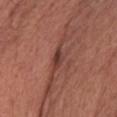follow-up = no biopsy performed (imaged during a skin exam) | diameter = ≈3 mm | imaging modality = ~15 mm crop, total-body skin-cancer survey | automated lesion analysis = a footprint of about 3.5 mm² and two-axis asymmetry of about 0.2; a nevus-likeness score of about 10/100 | illumination = white-light | subject = female, aged 63 to 67 | site = the chest.